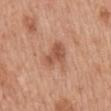Part of a total-body skin-imaging series; this lesion was reviewed on a skin check and was not flagged for biopsy. Measured at roughly 3.5 mm in maximum diameter. The tile uses white-light illumination. A female subject approximately 40 years of age. On the mid back. The lesion-visualizer software estimated an area of roughly 6.5 mm², a shape eccentricity near 0.75, and a shape-asymmetry score of about 0.3 (0 = symmetric). The analysis additionally found an average lesion color of about L≈54 a*≈24 b*≈32 (CIELAB), roughly 10 lightness units darker than nearby skin, and a normalized lesion–skin contrast near 6.5. The software also gave a border-irregularity index near 3/10, a within-lesion color-variation index near 3/10, and peripheral color asymmetry of about 1. A close-up tile cropped from a whole-body skin photograph, about 15 mm across.